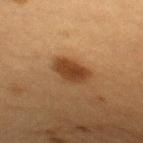<lesion>
  <image>
    <source>total-body photography crop</source>
    <field_of_view_mm>15</field_of_view_mm>
  </image>
  <site>upper back</site>
  <patient>
    <sex>female</sex>
    <age_approx>40</age_approx>
  </patient>
  <lesion_size>
    <long_diameter_mm_approx>4.5</long_diameter_mm_approx>
  </lesion_size>
  <automated_metrics>
    <cielab_L>34</cielab_L>
    <cielab_a>18</cielab_a>
    <cielab_b>30</cielab_b>
    <vs_skin_darker_L>11.0</vs_skin_darker_L>
    <vs_skin_contrast_norm>9.5</vs_skin_contrast_norm>
    <border_irregularity_0_10>2.5</border_irregularity_0_10>
    <color_variation_0_10>3.0</color_variation_0_10>
    <peripheral_color_asymmetry>1.0</peripheral_color_asymmetry>
  </automated_metrics>
</lesion>